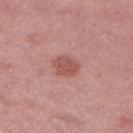The lesion was photographed on a routine skin check and not biopsied; there is no pathology result. The recorded lesion diameter is about 3 mm. The lesion is on the left thigh. The total-body-photography lesion software estimated an area of roughly 6.5 mm², an eccentricity of roughly 0.6, and two-axis asymmetry of about 0.15. It also reported a lesion color around L≈53 a*≈26 b*≈25 in CIELAB, about 10 CIELAB-L* units darker than the surrounding skin, and a normalized border contrast of about 7. And it measured lesion-presence confidence of about 100/100. Cropped from a whole-body photographic skin survey; the tile spans about 15 mm. The tile uses white-light illumination. The subject is a female in their 40s.